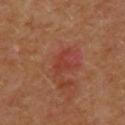Approximately 3.5 mm at its widest. A female subject, approximately 40 years of age. A roughly 15 mm field-of-view crop from a total-body skin photograph. The lesion is located on the upper back. Automated tile analysis of the lesion measured a lesion area of about 4 mm², an eccentricity of roughly 0.85, and a shape-asymmetry score of about 0.4 (0 = symmetric).